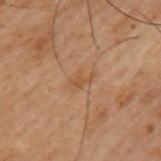biopsy_status: not biopsied; imaged during a skin examination
automated_metrics:
  vs_skin_darker_L: 5.0
  vs_skin_contrast_norm: 5.0
  border_irregularity_0_10: 5.0
  color_variation_0_10: 1.0
  peripheral_color_asymmetry: 0.5
site: left upper arm
patient:
  sex: male
  age_approx: 60
lesion_size:
  long_diameter_mm_approx: 3.0
lighting: cross-polarized
image:
  source: total-body photography crop
  field_of_view_mm: 15